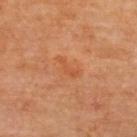Case summary:
• notes: imaged on a skin check; not biopsied
• lesion size: about 3 mm
• subject: female, about 65 years old
• body site: the back
• acquisition: ~15 mm tile from a whole-body skin photo
• tile lighting: cross-polarized illumination